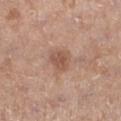Part of a total-body skin-imaging series; this lesion was reviewed on a skin check and was not flagged for biopsy.
The lesion is on the left lower leg.
The lesion-visualizer software estimated a classifier nevus-likeness of about 55/100.
A female patient aged 38–42.
A region of skin cropped from a whole-body photographic capture, roughly 15 mm wide.
The lesion's longest dimension is about 3 mm.